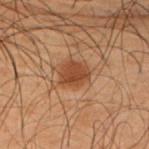Recorded during total-body skin imaging; not selected for excision or biopsy.
A male subject approximately 50 years of age.
The lesion's longest dimension is about 3 mm.
The lesion-visualizer software estimated radial color variation of about 0.5.
Captured under cross-polarized illumination.
Cropped from a total-body skin-imaging series; the visible field is about 15 mm.
The lesion is on the arm.